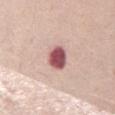Part of a total-body skin-imaging series; this lesion was reviewed on a skin check and was not flagged for biopsy. Cropped from a total-body skin-imaging series; the visible field is about 15 mm. Located on the chest. The total-body-photography lesion software estimated border irregularity of about 1.5 on a 0–10 scale, a within-lesion color-variation index near 4/10, and peripheral color asymmetry of about 1. A female subject aged 63–67. Measured at roughly 3.5 mm in maximum diameter.